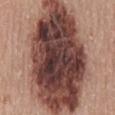This lesion was catalogued during total-body skin photography and was not selected for biopsy.
A lesion tile, about 15 mm wide, cut from a 3D total-body photograph.
Located on the back.
The lesion's longest dimension is about 15.5 mm.
The tile uses white-light illumination.
Automated tile analysis of the lesion measured a shape eccentricity near 0.85 and a symmetry-axis asymmetry near 0.2. It also reported a mean CIELAB color near L≈42 a*≈21 b*≈22 and about 21 CIELAB-L* units darker than the surrounding skin.
A male patient, aged around 60.